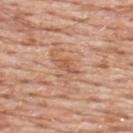Case summary:
• biopsy status · no biopsy performed (imaged during a skin exam)
• anatomic site · the upper back
• patient · male, aged around 60
• size · ≈2.5 mm
• tile lighting · white-light
• acquisition · ~15 mm crop, total-body skin-cancer survey